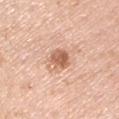Q: What is the imaging modality?
A: total-body-photography crop, ~15 mm field of view
Q: Where on the body is the lesion?
A: the left upper arm
Q: Who is the patient?
A: male, roughly 40 years of age
Q: How was the tile lit?
A: white-light illumination